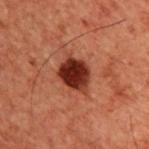An algorithmic analysis of the crop reported an area of roughly 9.5 mm², a shape eccentricity near 0.6, and two-axis asymmetry of about 0.2. It also reported a mean CIELAB color near L≈24 a*≈24 b*≈24 and about 15 CIELAB-L* units darker than the surrounding skin. About 4 mm across. A male subject, aged 58–62. A 15 mm close-up extracted from a 3D total-body photography capture. Located on the back.